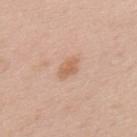The lesion was photographed on a routine skin check and not biopsied; there is no pathology result.
A male patient aged approximately 70.
Imaged with white-light lighting.
A lesion tile, about 15 mm wide, cut from a 3D total-body photograph.
Measured at roughly 3 mm in maximum diameter.
The lesion is located on the upper back.